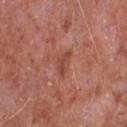Q: Was a biopsy performed?
A: imaged on a skin check; not biopsied
Q: What kind of image is this?
A: total-body-photography crop, ~15 mm field of view
Q: Patient demographics?
A: male, aged approximately 65
Q: Lesion size?
A: about 3 mm
Q: Where on the body is the lesion?
A: the chest
Q: How was the tile lit?
A: white-light illumination